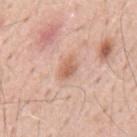Recorded during total-body skin imaging; not selected for excision or biopsy. On the mid back. A male subject aged 58 to 62. This image is a 15 mm lesion crop taken from a total-body photograph.